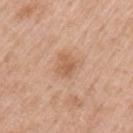follow-up: imaged on a skin check; not biopsied | subject: male, aged approximately 60 | location: the left upper arm | acquisition: total-body-photography crop, ~15 mm field of view.